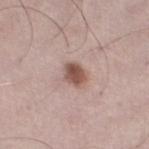– notes: total-body-photography surveillance lesion; no biopsy
– subject: male, aged approximately 50
– size: ≈2.5 mm
– site: the leg
– image source: total-body-photography crop, ~15 mm field of view